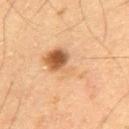Q: Is there a histopathology result?
A: catalogued during a skin exam; not biopsied
Q: What are the patient's age and sex?
A: male, about 60 years old
Q: What is the lesion's diameter?
A: ~8 mm (longest diameter)
Q: Automated lesion metrics?
A: an area of roughly 19 mm², a shape eccentricity near 0.85, and a shape-asymmetry score of about 0.45 (0 = symmetric); a lesion–skin lightness drop of about 8 and a lesion-to-skin contrast of about 5.5 (normalized; higher = more distinct); an automated nevus-likeness rating near 100 out of 100 and a lesion-detection confidence of about 100/100
Q: What is the imaging modality?
A: ~15 mm tile from a whole-body skin photo
Q: Illumination type?
A: cross-polarized illumination